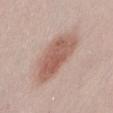<lesion>
<biopsy_status>not biopsied; imaged during a skin examination</biopsy_status>
<site>front of the torso</site>
<lesion_size>
  <long_diameter_mm_approx>7.0</long_diameter_mm_approx>
</lesion_size>
<lighting>white-light</lighting>
<image>
  <source>total-body photography crop</source>
  <field_of_view_mm>15</field_of_view_mm>
</image>
<patient>
  <sex>female</sex>
  <age_approx>30</age_approx>
</patient>
</lesion>